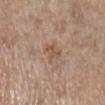Assessment:
Recorded during total-body skin imaging; not selected for excision or biopsy.
Context:
On the right lower leg. An algorithmic analysis of the crop reported a border-irregularity rating of about 4.5/10, internal color variation of about 0 on a 0–10 scale, and peripheral color asymmetry of about 0. It also reported an automated nevus-likeness rating near 0 out of 100 and lesion-presence confidence of about 100/100. About 2.5 mm across. This is a white-light tile. A 15 mm crop from a total-body photograph taken for skin-cancer surveillance. A female subject, aged approximately 60.